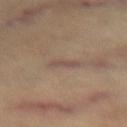{
  "biopsy_status": "not biopsied; imaged during a skin examination",
  "patient": {
    "sex": "female",
    "age_approx": 65
  },
  "automated_metrics": {
    "cielab_L": 47,
    "cielab_a": 15,
    "cielab_b": 22,
    "vs_skin_darker_L": 6.0,
    "vs_skin_contrast_norm": 6.0
  },
  "lesion_size": {
    "long_diameter_mm_approx": 3.0
  },
  "image": {
    "source": "total-body photography crop",
    "field_of_view_mm": 15
  },
  "lighting": "cross-polarized",
  "site": "left thigh"
}